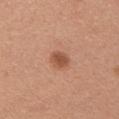Case summary:
- tile lighting: white-light illumination
- site: the chest
- patient: male, about 30 years old
- imaging modality: 15 mm crop, total-body photography